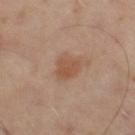This lesion was catalogued during total-body skin photography and was not selected for biopsy.
Automated tile analysis of the lesion measured a lesion area of about 6.5 mm² and a symmetry-axis asymmetry near 0.25. The analysis additionally found a nevus-likeness score of about 65/100.
The tile uses cross-polarized illumination.
About 3 mm across.
A male patient about 50 years old.
A close-up tile cropped from a whole-body skin photograph, about 15 mm across.
The lesion is on the left leg.